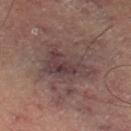No biopsy was performed on this lesion — it was imaged during a full skin examination and was not determined to be concerning. Imaged with cross-polarized lighting. The lesion is on the left thigh. A roughly 15 mm field-of-view crop from a total-body skin photograph. A male patient, in their mid-60s. Automated image analysis of the tile measured a shape eccentricity near 0.85. The analysis additionally found an average lesion color of about L≈39 a*≈17 b*≈16 (CIELAB) and a normalized border contrast of about 7.5. And it measured a nevus-likeness score of about 5/100 and a lesion-detection confidence of about 70/100.